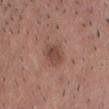No biopsy was performed on this lesion — it was imaged during a full skin examination and was not determined to be concerning. From the front of the torso. This is a white-light tile. A male patient, aged approximately 25. A lesion tile, about 15 mm wide, cut from a 3D total-body photograph. The recorded lesion diameter is about 3 mm.